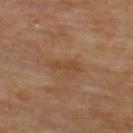  patient:
    sex: male
    age_approx: 70
  lesion_size:
    long_diameter_mm_approx: 3.5
  site: upper back
  lighting: cross-polarized
  image:
    source: total-body photography crop
    field_of_view_mm: 15